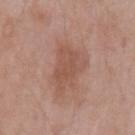follow-up — total-body-photography surveillance lesion; no biopsy
automated lesion analysis — an outline eccentricity of about 0.55 (0 = round, 1 = elongated) and a symmetry-axis asymmetry near 0.4; a lesion color around L≈53 a*≈21 b*≈27 in CIELAB and a normalized border contrast of about 5.5; a nevus-likeness score of about 0/100 and a lesion-detection confidence of about 100/100
body site — the left upper arm
image source — ~15 mm tile from a whole-body skin photo
lighting — white-light
lesion diameter — ~5 mm (longest diameter)
patient — male, aged 63 to 67